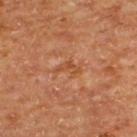| feature | finding |
|---|---|
| follow-up | catalogued during a skin exam; not biopsied |
| illumination | cross-polarized |
| patient | male, aged 63–67 |
| imaging modality | ~15 mm crop, total-body skin-cancer survey |
| size | ≈3 mm |
| body site | the upper back |